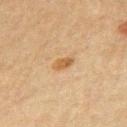A close-up tile cropped from a whole-body skin photograph, about 15 mm across. Measured at roughly 2.5 mm in maximum diameter. On the chest. A male subject in their 50s. The total-body-photography lesion software estimated a mean CIELAB color near L≈47 a*≈16 b*≈34, a lesion–skin lightness drop of about 8, and a normalized lesion–skin contrast near 7.5. It also reported a color-variation rating of about 2/10 and a peripheral color-asymmetry measure near 0.5. The software also gave a classifier nevus-likeness of about 65/100 and a lesion-detection confidence of about 100/100.